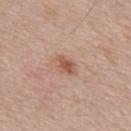image: ~15 mm tile from a whole-body skin photo | lesion diameter: ~3 mm (longest diameter) | patient: male, in their mid- to late 50s | image-analysis metrics: an average lesion color of about L≈55 a*≈20 b*≈30 (CIELAB), about 10 CIELAB-L* units darker than the surrounding skin, and a lesion-to-skin contrast of about 7.5 (normalized; higher = more distinct); border irregularity of about 3 on a 0–10 scale and a color-variation rating of about 3/10; a nevus-likeness score of about 70/100 and lesion-presence confidence of about 100/100 | lighting: white-light illumination.